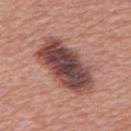This lesion was catalogued during total-body skin photography and was not selected for biopsy. The lesion is on the mid back. The tile uses white-light illumination. A male subject, about 65 years old. The recorded lesion diameter is about 8 mm. This image is a 15 mm lesion crop taken from a total-body photograph. The lesion-visualizer software estimated a lesion-detection confidence of about 100/100.